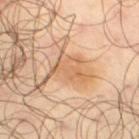Clinical impression: The lesion was photographed on a routine skin check and not biopsied; there is no pathology result. Context: From the leg. Imaged with cross-polarized lighting. A 15 mm close-up tile from a total-body photography series done for melanoma screening. A male subject, in their mid- to late 60s.